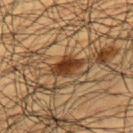| key | value |
|---|---|
| follow-up | total-body-photography surveillance lesion; no biopsy |
| patient | male, aged approximately 60 |
| image source | total-body-photography crop, ~15 mm field of view |
| lighting | cross-polarized illumination |
| lesion size | ≈3.5 mm |
| location | the left upper arm |